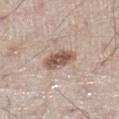Case summary:
– notes — no biopsy performed (imaged during a skin exam)
– diameter — about 3.5 mm
– subject — male, aged approximately 60
– anatomic site — the left lower leg
– tile lighting — white-light illumination
– acquisition — 15 mm crop, total-body photography